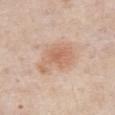Findings:
• biopsy status · no biopsy performed (imaged during a skin exam)
• imaging modality · 15 mm crop, total-body photography
• lighting · white-light
• subject · male, approximately 85 years of age or older
• lesion size · ≈5 mm
• body site · the abdomen